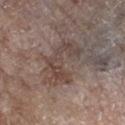Captured during whole-body skin photography for melanoma surveillance; the lesion was not biopsied. The lesion is located on the left lower leg. A lesion tile, about 15 mm wide, cut from a 3D total-body photograph. Automated tile analysis of the lesion measured a lesion area of about 14 mm², a shape eccentricity near 0.85, and a shape-asymmetry score of about 0.55 (0 = symmetric). The tile uses white-light illumination. A female subject, aged 83 or older.